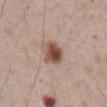  biopsy_status: not biopsied; imaged during a skin examination
  patient:
    sex: male
    age_approx: 65
  image:
    source: total-body photography crop
    field_of_view_mm: 15
  automated_metrics:
    area_mm2_approx: 7.5
    eccentricity: 0.55
    shape_asymmetry: 0.2
    cielab_L: 50
    cielab_a: 19
    cielab_b: 26
    vs_skin_contrast_norm: 10.5
    nevus_likeness_0_100: 100
    lesion_detection_confidence_0_100: 100
  site: abdomen
  lesion_size:
    long_diameter_mm_approx: 3.0
  lighting: white-light